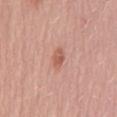notes: catalogued during a skin exam; not biopsied
patient: male, aged 53 to 57
acquisition: total-body-photography crop, ~15 mm field of view
diameter: ≈2.5 mm
site: the mid back
lighting: white-light illumination